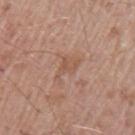Impression:
Part of a total-body skin-imaging series; this lesion was reviewed on a skin check and was not flagged for biopsy.
Background:
This image is a 15 mm lesion crop taken from a total-body photograph. A male subject roughly 70 years of age. An algorithmic analysis of the crop reported a border-irregularity rating of about 6/10 and radial color variation of about 0.5. It also reported an automated nevus-likeness rating near 0 out of 100 and a detector confidence of about 100 out of 100 that the crop contains a lesion. The lesion is on the left upper arm.